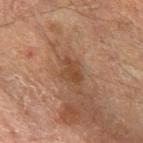workup: no biopsy performed (imaged during a skin exam)
location: the arm
lighting: cross-polarized illumination
image: total-body-photography crop, ~15 mm field of view
patient: male, aged around 65